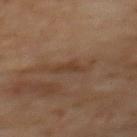| feature | finding |
|---|---|
| follow-up | catalogued during a skin exam; not biopsied |
| automated lesion analysis | a lesion area of about 2.5 mm², an outline eccentricity of about 0.9 (0 = round, 1 = elongated), and two-axis asymmetry of about 0.5; a mean CIELAB color near L≈37 a*≈18 b*≈28, roughly 6 lightness units darker than nearby skin, and a normalized border contrast of about 6; a classifier nevus-likeness of about 0/100 and lesion-presence confidence of about 100/100 |
| image | ~15 mm tile from a whole-body skin photo |
| site | the mid back |
| illumination | cross-polarized |
| size | ~2.5 mm (longest diameter) |
| subject | male, aged 68–72 |